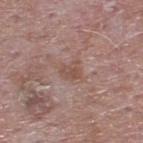{
  "biopsy_status": "not biopsied; imaged during a skin examination",
  "patient": {
    "sex": "male",
    "age_approx": 65
  },
  "image": {
    "source": "total-body photography crop",
    "field_of_view_mm": 15
  },
  "site": "upper back"
}